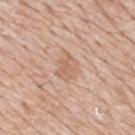On the mid back. Measured at roughly 3.5 mm in maximum diameter. The patient is a male about 80 years old. A lesion tile, about 15 mm wide, cut from a 3D total-body photograph. Imaged with white-light lighting.